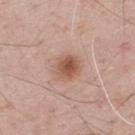Q: Was a biopsy performed?
A: no biopsy performed (imaged during a skin exam)
Q: How was this image acquired?
A: 15 mm crop, total-body photography
Q: Lesion location?
A: the upper back
Q: Patient demographics?
A: male, roughly 70 years of age